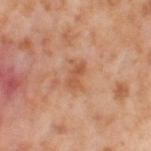The lesion was photographed on a routine skin check and not biopsied; there is no pathology result. A region of skin cropped from a whole-body photographic capture, roughly 15 mm wide. This is a cross-polarized tile. The recorded lesion diameter is about 3 mm. On the right thigh. The patient is a female roughly 55 years of age.